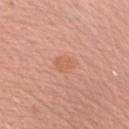No biopsy was performed on this lesion — it was imaged during a full skin examination and was not determined to be concerning. A 15 mm close-up tile from a total-body photography series done for melanoma screening. Automated image analysis of the tile measured an average lesion color of about L≈61 a*≈24 b*≈33 (CIELAB), about 6 CIELAB-L* units darker than the surrounding skin, and a lesion-to-skin contrast of about 4.5 (normalized; higher = more distinct). And it measured internal color variation of about 1.5 on a 0–10 scale and peripheral color asymmetry of about 0.5. The recorded lesion diameter is about 2.5 mm. From the right upper arm. The subject is a female aged 43–47. The tile uses white-light illumination.